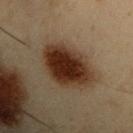{
  "biopsy_status": "not biopsied; imaged during a skin examination",
  "patient": {
    "sex": "male",
    "age_approx": 55
  },
  "lighting": "cross-polarized",
  "automated_metrics": {
    "cielab_L": 25,
    "cielab_a": 14,
    "cielab_b": 23,
    "vs_skin_darker_L": 13.0,
    "vs_skin_contrast_norm": 13.5,
    "border_irregularity_0_10": 1.5,
    "color_variation_0_10": 5.5,
    "peripheral_color_asymmetry": 1.5,
    "nevus_likeness_0_100": 100,
    "lesion_detection_confidence_0_100": 100
  },
  "lesion_size": {
    "long_diameter_mm_approx": 6.0
  },
  "site": "left upper arm",
  "image": {
    "source": "total-body photography crop",
    "field_of_view_mm": 15
  }
}